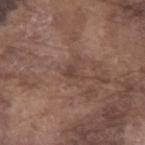Q: Is there a histopathology result?
A: imaged on a skin check; not biopsied
Q: What is the lesion's diameter?
A: ≈2.5 mm
Q: Automated lesion metrics?
A: a classifier nevus-likeness of about 0/100
Q: How was this image acquired?
A: 15 mm crop, total-body photography
Q: Who is the patient?
A: male, aged around 75
Q: What is the anatomic site?
A: the left forearm
Q: What lighting was used for the tile?
A: white-light